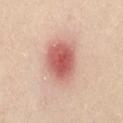Assessment:
No biopsy was performed on this lesion — it was imaged during a full skin examination and was not determined to be concerning.
Background:
From the abdomen. Cropped from a whole-body photographic skin survey; the tile spans about 15 mm. The lesion's longest dimension is about 5 mm. The tile uses cross-polarized illumination. A female patient, roughly 40 years of age. The total-body-photography lesion software estimated an area of roughly 17 mm², an outline eccentricity of about 0.65 (0 = round, 1 = elongated), and two-axis asymmetry of about 0.15. The software also gave a mean CIELAB color near L≈60 a*≈28 b*≈27, a lesion–skin lightness drop of about 15, and a normalized border contrast of about 9.